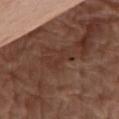Assessment:
Captured during whole-body skin photography for melanoma surveillance; the lesion was not biopsied.
Background:
Located on the abdomen. This image is a 15 mm lesion crop taken from a total-body photograph. A female patient roughly 75 years of age.